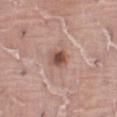follow-up: imaged on a skin check; not biopsied
diameter: about 2.5 mm
anatomic site: the front of the torso
lighting: white-light
image-analysis metrics: a shape eccentricity near 0.35 and two-axis asymmetry of about 0.2; an automated nevus-likeness rating near 85 out of 100 and lesion-presence confidence of about 100/100
imaging modality: ~15 mm crop, total-body skin-cancer survey
subject: male, aged around 40